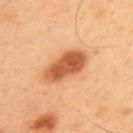Clinical impression:
No biopsy was performed on this lesion — it was imaged during a full skin examination and was not determined to be concerning.
Clinical summary:
Approximately 5.5 mm at its widest. The subject is a male aged 53 to 57. Captured under cross-polarized illumination. Cropped from a whole-body photographic skin survey; the tile spans about 15 mm. Located on the back.